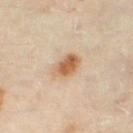Q: Was this lesion biopsied?
A: no biopsy performed (imaged during a skin exam)
Q: How was the tile lit?
A: cross-polarized
Q: What are the patient's age and sex?
A: female, about 60 years old
Q: What is the anatomic site?
A: the right thigh
Q: Lesion size?
A: ~3.5 mm (longest diameter)
Q: How was this image acquired?
A: total-body-photography crop, ~15 mm field of view